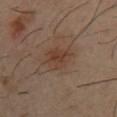workup = catalogued during a skin exam; not biopsied
image = ~15 mm tile from a whole-body skin photo
anatomic site = the chest
patient = male, aged 38 to 42